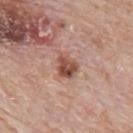  biopsy_status: not biopsied; imaged during a skin examination
  site: mid back
  patient:
    sex: male
    age_approx: 80
  lesion_size:
    long_diameter_mm_approx: 3.0
  image:
    source: total-body photography crop
    field_of_view_mm: 15
  lighting: white-light
  automated_metrics:
    area_mm2_approx: 6.0
    cielab_L: 50
    cielab_a: 23
    cielab_b: 28
    vs_skin_darker_L: 14.0
    vs_skin_contrast_norm: 9.5
    border_irregularity_0_10: 2.0
    color_variation_0_10: 6.0
    peripheral_color_asymmetry: 2.5
    lesion_detection_confidence_0_100: 100